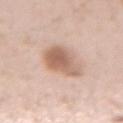Case summary:
– workup — no biopsy performed (imaged during a skin exam)
– image source — ~15 mm tile from a whole-body skin photo
– location — the left forearm
– diameter — ≈4 mm
– subject — female, about 20 years old
– image-analysis metrics — a footprint of about 13 mm², an eccentricity of roughly 0.4, and a shape-asymmetry score of about 0.25 (0 = symmetric)